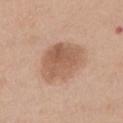biopsy status: imaged on a skin check; not biopsied | body site: the chest | lighting: white-light | imaging modality: 15 mm crop, total-body photography | patient: male, aged approximately 60 | lesion size: about 6 mm | automated lesion analysis: a lesion area of about 21 mm²; about 10 CIELAB-L* units darker than the surrounding skin and a normalized lesion–skin contrast near 7; a border-irregularity rating of about 2/10, a color-variation rating of about 4/10, and radial color variation of about 1.5; a nevus-likeness score of about 15/100 and a detector confidence of about 100 out of 100 that the crop contains a lesion.